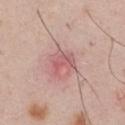{
  "biopsy_status": "not biopsied; imaged during a skin examination",
  "lighting": "white-light",
  "lesion_size": {
    "long_diameter_mm_approx": 3.0
  },
  "image": {
    "source": "total-body photography crop",
    "field_of_view_mm": 15
  },
  "patient": {
    "sex": "male",
    "age_approx": 60
  },
  "site": "chest"
}